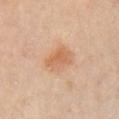Assessment: Part of a total-body skin-imaging series; this lesion was reviewed on a skin check and was not flagged for biopsy. Background: Automated image analysis of the tile measured a nevus-likeness score of about 80/100 and a lesion-detection confidence of about 100/100. A female subject, roughly 60 years of age. Captured under cross-polarized illumination. From the chest. A 15 mm crop from a total-body photograph taken for skin-cancer surveillance. Approximately 3.5 mm at its widest.